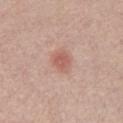notes: no biopsy performed (imaged during a skin exam)
image source: total-body-photography crop, ~15 mm field of view
body site: the front of the torso
subject: female, about 70 years old